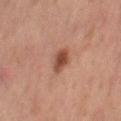<lesion>
  <biopsy_status>not biopsied; imaged during a skin examination</biopsy_status>
  <image>
    <source>total-body photography crop</source>
    <field_of_view_mm>15</field_of_view_mm>
  </image>
  <patient>
    <sex>female</sex>
    <age_approx>60</age_approx>
  </patient>
  <lesion_size>
    <long_diameter_mm_approx>3.0</long_diameter_mm_approx>
  </lesion_size>
  <site>leg</site>
</lesion>